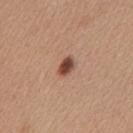Assessment: Recorded during total-body skin imaging; not selected for excision or biopsy. Acquisition and patient details: The lesion is on the chest. Cropped from a whole-body photographic skin survey; the tile spans about 15 mm. The patient is a female approximately 45 years of age.